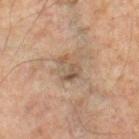Notes:
– biopsy status — catalogued during a skin exam; not biopsied
– subject — male, about 45 years old
– site — the right lower leg
– lighting — cross-polarized illumination
– acquisition — total-body-photography crop, ~15 mm field of view
– lesion size — ~3.5 mm (longest diameter)